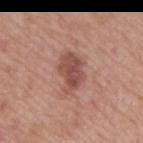Clinical impression:
The lesion was photographed on a routine skin check and not biopsied; there is no pathology result.
Background:
A male subject aged 73 to 77. On the mid back. A region of skin cropped from a whole-body photographic capture, roughly 15 mm wide.